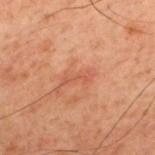Assessment: The lesion was tiled from a total-body skin photograph and was not biopsied. Clinical summary: This is a cross-polarized tile. The lesion-visualizer software estimated an area of roughly 4.5 mm². The software also gave an average lesion color of about L≈43 a*≈23 b*≈27 (CIELAB) and a lesion-to-skin contrast of about 4.5 (normalized; higher = more distinct). It also reported a border-irregularity index near 4/10, internal color variation of about 2 on a 0–10 scale, and radial color variation of about 0.5. And it measured a classifier nevus-likeness of about 0/100 and a lesion-detection confidence of about 100/100. The recorded lesion diameter is about 3.5 mm. A 15 mm crop from a total-body photograph taken for skin-cancer surveillance. A male patient aged 58 to 62. The lesion is located on the back.